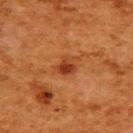No biopsy was performed on this lesion — it was imaged during a full skin examination and was not determined to be concerning. The lesion's longest dimension is about 2.5 mm. The patient is a female aged around 50. On the back. The total-body-photography lesion software estimated a lesion area of about 4.5 mm², an outline eccentricity of about 0.55 (0 = round, 1 = elongated), and two-axis asymmetry of about 0.35. A roughly 15 mm field-of-view crop from a total-body skin photograph. Captured under cross-polarized illumination.